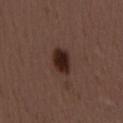The lesion was photographed on a routine skin check and not biopsied; there is no pathology result.
A female subject, aged 48 to 52.
This is a white-light tile.
The lesion is located on the back.
The lesion's longest dimension is about 3.5 mm.
Automated image analysis of the tile measured a footprint of about 7.5 mm² and a shape-asymmetry score of about 0.15 (0 = symmetric). It also reported an average lesion color of about L≈26 a*≈17 b*≈20 (CIELAB), roughly 12 lightness units darker than nearby skin, and a normalized lesion–skin contrast near 12. It also reported an automated nevus-likeness rating near 100 out of 100.
A close-up tile cropped from a whole-body skin photograph, about 15 mm across.